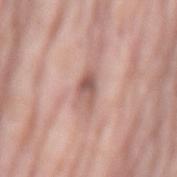Imaged during a routine full-body skin examination; the lesion was not biopsied and no histopathology is available. This is a white-light tile. About 3.5 mm across. The lesion is on the mid back. A roughly 15 mm field-of-view crop from a total-body skin photograph. A female subject, roughly 75 years of age.